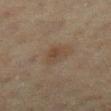Part of a total-body skin-imaging series; this lesion was reviewed on a skin check and was not flagged for biopsy.